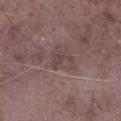Assessment:
Captured during whole-body skin photography for melanoma surveillance; the lesion was not biopsied.
Context:
The lesion is located on the left lower leg. Cropped from a whole-body photographic skin survey; the tile spans about 15 mm. Imaged with white-light lighting. The subject is a male in their mid- to late 70s.